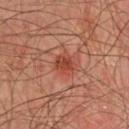No biopsy was performed on this lesion — it was imaged during a full skin examination and was not determined to be concerning. Located on the chest. Longest diameter approximately 3 mm. Automated tile analysis of the lesion measured a footprint of about 5.5 mm², a shape eccentricity near 0.55, and a shape-asymmetry score of about 0.3 (0 = symmetric). And it measured a nevus-likeness score of about 100/100. A male subject roughly 65 years of age. Imaged with cross-polarized lighting. A close-up tile cropped from a whole-body skin photograph, about 15 mm across.